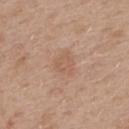Impression:
Captured during whole-body skin photography for melanoma surveillance; the lesion was not biopsied.
Background:
On the upper back. Cropped from a total-body skin-imaging series; the visible field is about 15 mm. A female subject in their 40s.